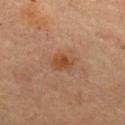Q: Is there a histopathology result?
A: catalogued during a skin exam; not biopsied
Q: What is the lesion's diameter?
A: ≈2.5 mm
Q: Who is the patient?
A: female, aged around 60
Q: Illumination type?
A: cross-polarized illumination
Q: What is the imaging modality?
A: ~15 mm tile from a whole-body skin photo
Q: What did automated image analysis measure?
A: a within-lesion color-variation index near 3.5/10
Q: Where on the body is the lesion?
A: the chest